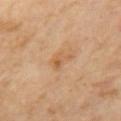Assessment:
Part of a total-body skin-imaging series; this lesion was reviewed on a skin check and was not flagged for biopsy.
Background:
Automated image analysis of the tile measured a footprint of about 3 mm², an eccentricity of roughly 0.75, and a shape-asymmetry score of about 0.6 (0 = symmetric). And it measured a border-irregularity index near 6.5/10, internal color variation of about 2.5 on a 0–10 scale, and radial color variation of about 1. This is a cross-polarized tile. Cropped from a whole-body photographic skin survey; the tile spans about 15 mm. Longest diameter approximately 2.5 mm. Located on the mid back.